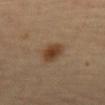A female patient aged approximately 60. This is a cross-polarized tile. About 3 mm across. A 15 mm close-up extracted from a 3D total-body photography capture. The lesion-visualizer software estimated an average lesion color of about L≈42 a*≈18 b*≈31 (CIELAB) and a normalized lesion–skin contrast near 9. From the abdomen.